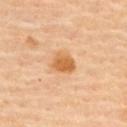The subject is a female aged approximately 60.
The lesion is located on the back.
This is a cross-polarized tile.
Approximately 3 mm at its widest.
Automated tile analysis of the lesion measured a shape eccentricity near 0.6 and a shape-asymmetry score of about 0.25 (0 = symmetric). It also reported a lesion–skin lightness drop of about 12 and a lesion-to-skin contrast of about 8.5 (normalized; higher = more distinct). And it measured a within-lesion color-variation index near 3.5/10 and a peripheral color-asymmetry measure near 1. The software also gave an automated nevus-likeness rating near 95 out of 100 and lesion-presence confidence of about 100/100.
A region of skin cropped from a whole-body photographic capture, roughly 15 mm wide.